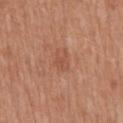Captured during whole-body skin photography for melanoma surveillance; the lesion was not biopsied. On the mid back. Automated image analysis of the tile measured an area of roughly 4 mm², an outline eccentricity of about 0.7 (0 = round, 1 = elongated), and two-axis asymmetry of about 0.4. And it measured an average lesion color of about L≈52 a*≈24 b*≈32 (CIELAB), roughly 6 lightness units darker than nearby skin, and a lesion-to-skin contrast of about 4.5 (normalized; higher = more distinct). The software also gave a border-irregularity rating of about 5/10, a color-variation rating of about 1/10, and a peripheral color-asymmetry measure near 0.5. And it measured a nevus-likeness score of about 0/100 and a detector confidence of about 100 out of 100 that the crop contains a lesion. Cropped from a whole-body photographic skin survey; the tile spans about 15 mm. Imaged with white-light lighting. The patient is a male aged 68–72.